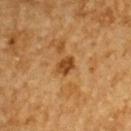Image and clinical context:
From the upper back. Measured at roughly 2.5 mm in maximum diameter. The patient is a male in their mid-80s or older. A roughly 15 mm field-of-view crop from a total-body skin photograph. The tile uses cross-polarized illumination.